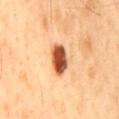The lesion was tiled from a total-body skin photograph and was not biopsied. On the mid back. A 15 mm close-up extracted from a 3D total-body photography capture. A male patient, about 45 years old. About 4 mm across.